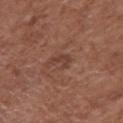Impression:
The lesion was photographed on a routine skin check and not biopsied; there is no pathology result.
Acquisition and patient details:
Located on the chest. A female patient aged approximately 75. A roughly 15 mm field-of-view crop from a total-body skin photograph.